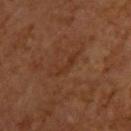workup: total-body-photography surveillance lesion; no biopsy
lighting: cross-polarized illumination
location: the upper back
patient: male, in their mid-60s
lesion diameter: ≈4.5 mm
image source: 15 mm crop, total-body photography